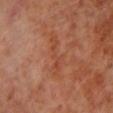Case summary:
– workup · imaged on a skin check; not biopsied
– acquisition · total-body-photography crop, ~15 mm field of view
– site · the left lower leg
– patient · male, roughly 70 years of age
– lesion diameter · about 5.5 mm
– TBP lesion metrics · a footprint of about 6.5 mm² and a symmetry-axis asymmetry near 0.6; a classifier nevus-likeness of about 0/100 and lesion-presence confidence of about 95/100